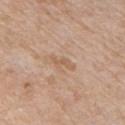Q: Was a biopsy performed?
A: catalogued during a skin exam; not biopsied
Q: Illumination type?
A: white-light illumination
Q: What is the imaging modality?
A: 15 mm crop, total-body photography
Q: Lesion location?
A: the chest
Q: What are the patient's age and sex?
A: male, aged approximately 65
Q: How large is the lesion?
A: about 3.5 mm